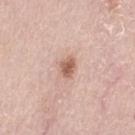Findings:
- body site — the left thigh
- subject — female, approximately 65 years of age
- lesion diameter — ~2.5 mm (longest diameter)
- illumination — white-light
- imaging modality — 15 mm crop, total-body photography